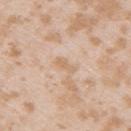Acquisition and patient details: The recorded lesion diameter is about 2.5 mm. A 15 mm crop from a total-body photograph taken for skin-cancer surveillance. A female patient aged around 25. On the right upper arm. The total-body-photography lesion software estimated a color-variation rating of about 0/10 and a peripheral color-asymmetry measure near 0. And it measured an automated nevus-likeness rating near 0 out of 100 and a detector confidence of about 100 out of 100 that the crop contains a lesion.